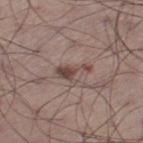<lesion>
<biopsy_status>not biopsied; imaged during a skin examination</biopsy_status>
<lighting>white-light</lighting>
<lesion_size>
  <long_diameter_mm_approx>4.0</long_diameter_mm_approx>
</lesion_size>
<patient>
  <sex>male</sex>
  <age_approx>55</age_approx>
</patient>
<image>
  <source>total-body photography crop</source>
  <field_of_view_mm>15</field_of_view_mm>
</image>
<site>left thigh</site>
</lesion>